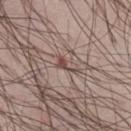notes: no biopsy performed (imaged during a skin exam) | subject: male, aged 48–52 | TBP lesion metrics: a lesion area of about 2.5 mm², an eccentricity of roughly 0.95, and two-axis asymmetry of about 0.55; a border-irregularity index near 6.5/10, a within-lesion color-variation index near 0/10, and radial color variation of about 0 | image source: ~15 mm tile from a whole-body skin photo | anatomic site: the right thigh | size: about 3 mm.